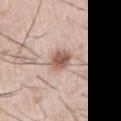notes: no biopsy performed (imaged during a skin exam)
subject: male, aged 53–57
acquisition: total-body-photography crop, ~15 mm field of view